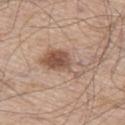This lesion was catalogued during total-body skin photography and was not selected for biopsy. The patient is a male about 60 years old. The lesion is on the left thigh. The lesion's longest dimension is about 6 mm. Cropped from a total-body skin-imaging series; the visible field is about 15 mm.